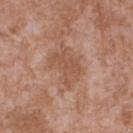Recorded during total-body skin imaging; not selected for excision or biopsy.
Automated image analysis of the tile measured an area of roughly 12 mm² and a shape eccentricity near 0.7. And it measured a lesion color around L≈53 a*≈21 b*≈30 in CIELAB. The software also gave lesion-presence confidence of about 100/100.
Approximately 4.5 mm at its widest.
A 15 mm close-up tile from a total-body photography series done for melanoma screening.
The tile uses white-light illumination.
The subject is a male aged approximately 45.
From the upper back.